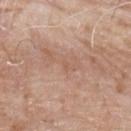Assessment: The lesion was tiled from a total-body skin photograph and was not biopsied. Background: This is a white-light tile. The lesion is on the upper back. A male subject, roughly 65 years of age. A close-up tile cropped from a whole-body skin photograph, about 15 mm across. The recorded lesion diameter is about 1 mm. Automated tile analysis of the lesion measured a nevus-likeness score of about 0/100 and lesion-presence confidence of about 100/100.